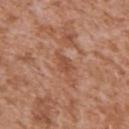Case summary:
- follow-up — imaged on a skin check; not biopsied
- image — total-body-photography crop, ~15 mm field of view
- subject — male, roughly 45 years of age
- anatomic site — the left upper arm